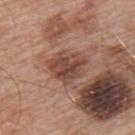Notes:
– follow-up: no biopsy performed (imaged during a skin exam)
– patient: male, in their mid- to late 50s
– acquisition: ~15 mm crop, total-body skin-cancer survey
– site: the upper back